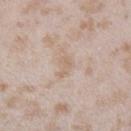<lesion>
<image>
  <source>total-body photography crop</source>
  <field_of_view_mm>15</field_of_view_mm>
</image>
<site>left lower leg</site>
<lesion_size>
  <long_diameter_mm_approx>2.5</long_diameter_mm_approx>
</lesion_size>
<patient>
  <sex>female</sex>
  <age_approx>25</age_approx>
</patient>
</lesion>